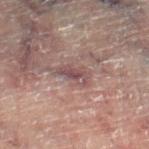* follow-up · no biopsy performed (imaged during a skin exam)
* diameter · ~3.5 mm (longest diameter)
* image · ~15 mm tile from a whole-body skin photo
* image-analysis metrics · a lesion–skin lightness drop of about 8; internal color variation of about 4 on a 0–10 scale and a peripheral color-asymmetry measure near 1
* subject · male, in their 60s
* site · the right lower leg
* illumination · cross-polarized illumination